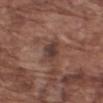Assessment: Imaged during a routine full-body skin examination; the lesion was not biopsied and no histopathology is available. Background: A 15 mm close-up extracted from a 3D total-body photography capture. Located on the right upper arm. The patient is a male aged around 75.